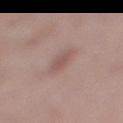{
  "biopsy_status": "not biopsied; imaged during a skin examination",
  "patient": {
    "sex": "female",
    "age_approx": 40
  },
  "site": "right lower leg",
  "image": {
    "source": "total-body photography crop",
    "field_of_view_mm": 15
  }
}